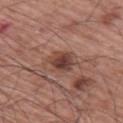Assessment: Recorded during total-body skin imaging; not selected for excision or biopsy. Context: A region of skin cropped from a whole-body photographic capture, roughly 15 mm wide. The lesion is on the right upper arm. The patient is a male aged approximately 65. About 3.5 mm across. Automated tile analysis of the lesion measured an average lesion color of about L≈43 a*≈21 b*≈25 (CIELAB), roughly 11 lightness units darker than nearby skin, and a normalized lesion–skin contrast near 8.5.